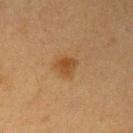Clinical impression: The lesion was tiled from a total-body skin photograph and was not biopsied. Context: A female patient about 40 years old. On the left upper arm. Automated image analysis of the tile measured an eccentricity of roughly 0.4 and a shape-asymmetry score of about 0.25 (0 = symmetric). The analysis additionally found a border-irregularity rating of about 2/10, a within-lesion color-variation index near 3/10, and a peripheral color-asymmetry measure near 1. A 15 mm close-up extracted from a 3D total-body photography capture. This is a cross-polarized tile. About 2.5 mm across.